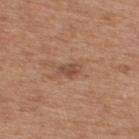Findings:
* follow-up: imaged on a skin check; not biopsied
* lighting: white-light
* patient: male, in their mid-50s
* diameter: ≈2.5 mm
* image source: 15 mm crop, total-body photography
* automated metrics: an area of roughly 3 mm² and a symmetry-axis asymmetry near 0.25; an average lesion color of about L≈49 a*≈21 b*≈29 (CIELAB), a lesion–skin lightness drop of about 9, and a normalized lesion–skin contrast near 6.5; a nevus-likeness score of about 0/100 and a detector confidence of about 100 out of 100 that the crop contains a lesion
* anatomic site: the upper back